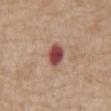biopsy_status: not biopsied; imaged during a skin examination
lesion_size:
  long_diameter_mm_approx: 3.0
patient:
  sex: male
  age_approx: 70
site: abdomen
lighting: white-light
image:
  source: total-body photography crop
  field_of_view_mm: 15